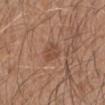Assessment:
Recorded during total-body skin imaging; not selected for excision or biopsy.
Acquisition and patient details:
Captured under white-light illumination. A roughly 15 mm field-of-view crop from a total-body skin photograph. An algorithmic analysis of the crop reported a lesion area of about 4 mm², an outline eccentricity of about 0.8 (0 = round, 1 = elongated), and two-axis asymmetry of about 0.25. The analysis additionally found about 7 CIELAB-L* units darker than the surrounding skin and a normalized border contrast of about 5.5. The software also gave border irregularity of about 3 on a 0–10 scale and a peripheral color-asymmetry measure near 0.5. The lesion is on the left forearm. The subject is a male aged 43–47. The recorded lesion diameter is about 2.5 mm.